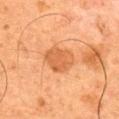The lesion was tiled from a total-body skin photograph and was not biopsied.
A male subject in their mid-60s.
The lesion is on the mid back.
A region of skin cropped from a whole-body photographic capture, roughly 15 mm wide.
The total-body-photography lesion software estimated an average lesion color of about L≈50 a*≈24 b*≈37 (CIELAB) and a normalized border contrast of about 6.5.
Approximately 4 mm at its widest.
This is a cross-polarized tile.